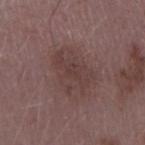Recorded during total-body skin imaging; not selected for excision or biopsy. The lesion is located on the right upper arm. A roughly 15 mm field-of-view crop from a total-body skin photograph. The lesion-visualizer software estimated an area of roughly 16 mm², an eccentricity of roughly 0.75, and a shape-asymmetry score of about 0.35 (0 = symmetric). The software also gave a mean CIELAB color near L≈39 a*≈17 b*≈18 and roughly 6 lightness units darker than nearby skin. The patient is a female roughly 45 years of age.